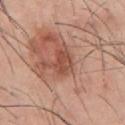Recorded during total-body skin imaging; not selected for excision or biopsy. From the chest. The patient is a male aged around 45. A lesion tile, about 15 mm wide, cut from a 3D total-body photograph.